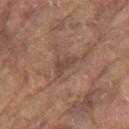workup: no biopsy performed (imaged during a skin exam)
body site: the left upper arm
lighting: white-light illumination
subject: male, aged 78 to 82
acquisition: total-body-photography crop, ~15 mm field of view